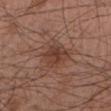anatomic site = the right forearm; imaging modality = ~15 mm tile from a whole-body skin photo; patient = male, in their mid- to late 50s; illumination = white-light; lesion size = ≈3.5 mm.